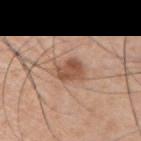The lesion was photographed on a routine skin check and not biopsied; there is no pathology result. Approximately 3 mm at its widest. Automated image analysis of the tile measured a footprint of about 6.5 mm², an outline eccentricity of about 0.6 (0 = round, 1 = elongated), and two-axis asymmetry of about 0.25. It also reported an average lesion color of about L≈52 a*≈21 b*≈31 (CIELAB), a lesion–skin lightness drop of about 12, and a normalized lesion–skin contrast near 8.5. The software also gave a border-irregularity rating of about 2.5/10, internal color variation of about 3 on a 0–10 scale, and radial color variation of about 1. It also reported a classifier nevus-likeness of about 80/100. This is a white-light tile. A roughly 15 mm field-of-view crop from a total-body skin photograph. The subject is a male in their 50s. Located on the right upper arm.